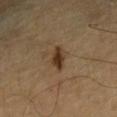biopsy status=total-body-photography surveillance lesion; no biopsy
patient=male, in their 60s
anatomic site=the front of the torso
image=~15 mm crop, total-body skin-cancer survey